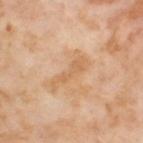Findings:
• lesion diameter: ≈5 mm
• body site: the left thigh
• automated metrics: an area of roughly 5 mm², a shape eccentricity near 0.95, and two-axis asymmetry of about 0.45; an average lesion color of about L≈64 a*≈21 b*≈38 (CIELAB), roughly 7 lightness units darker than nearby skin, and a normalized lesion–skin contrast near 5.5; a border-irregularity index near 7/10 and internal color variation of about 1 on a 0–10 scale; an automated nevus-likeness rating near 0 out of 100
• image source: 15 mm crop, total-body photography
• patient: female, roughly 55 years of age
• illumination: cross-polarized